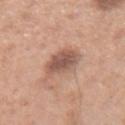Image and clinical context:
The subject is a female about 40 years old. A roughly 15 mm field-of-view crop from a total-body skin photograph. The lesion's longest dimension is about 4 mm. Imaged with white-light lighting. The lesion is located on the right forearm.